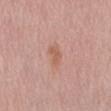workup: no biopsy performed (imaged during a skin exam) | patient: female, in their mid-60s | diameter: ≈2.5 mm | body site: the mid back | lighting: white-light | image: total-body-photography crop, ~15 mm field of view.